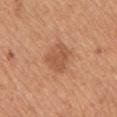Q: Was a biopsy performed?
A: imaged on a skin check; not biopsied
Q: What is the imaging modality?
A: total-body-photography crop, ~15 mm field of view
Q: What is the anatomic site?
A: the upper back
Q: Patient demographics?
A: female, aged around 45